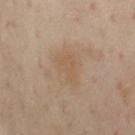The lesion was tiled from a total-body skin photograph and was not biopsied. A male patient, about 55 years old. The lesion-visualizer software estimated a footprint of about 6 mm², a shape eccentricity near 0.85, and a symmetry-axis asymmetry near 0.45. The analysis additionally found border irregularity of about 4.5 on a 0–10 scale and internal color variation of about 1 on a 0–10 scale. And it measured a lesion-detection confidence of about 100/100. About 4 mm across. A roughly 15 mm field-of-view crop from a total-body skin photograph. The lesion is located on the chest. The tile uses cross-polarized illumination.